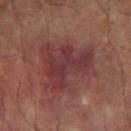workup: no biopsy performed (imaged during a skin exam) | size: ≈7 mm | patient: male, aged around 75 | imaging modality: 15 mm crop, total-body photography | location: the right forearm | tile lighting: cross-polarized illumination | image-analysis metrics: an area of roughly 28 mm², an eccentricity of roughly 0.6, and two-axis asymmetry of about 0.45; a classifier nevus-likeness of about 0/100 and lesion-presence confidence of about 95/100.